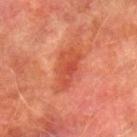biopsy_status: not biopsied; imaged during a skin examination
patient:
  sex: male
  age_approx: 75
lesion_size:
  long_diameter_mm_approx: 5.0
lighting: cross-polarized
image:
  source: total-body photography crop
  field_of_view_mm: 15
automated_metrics:
  cielab_L: 43
  cielab_a: 31
  cielab_b: 32
  vs_skin_contrast_norm: 6.5
  lesion_detection_confidence_0_100: 100
site: right lower leg